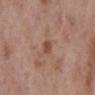Recorded during total-body skin imaging; not selected for excision or biopsy.
Cropped from a whole-body photographic skin survey; the tile spans about 15 mm.
A female subject aged 68 to 72.
About 2.5 mm across.
Automated tile analysis of the lesion measured an average lesion color of about L≈48 a*≈21 b*≈28 (CIELAB) and a normalized border contrast of about 7. And it measured a classifier nevus-likeness of about 0/100.
On the left lower leg.